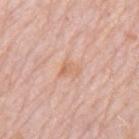Q: Was this lesion biopsied?
A: catalogued during a skin exam; not biopsied
Q: Illumination type?
A: white-light illumination
Q: What are the patient's age and sex?
A: male, roughly 80 years of age
Q: Lesion size?
A: about 2.5 mm
Q: Where on the body is the lesion?
A: the mid back
Q: Automated lesion metrics?
A: an area of roughly 3.5 mm² and a shape eccentricity near 0.7
Q: How was this image acquired?
A: total-body-photography crop, ~15 mm field of view